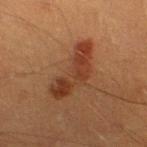Q: Was this lesion biopsied?
A: catalogued during a skin exam; not biopsied
Q: What lighting was used for the tile?
A: cross-polarized illumination
Q: Lesion location?
A: the left lower leg
Q: Automated lesion metrics?
A: a footprint of about 14 mm², a shape eccentricity near 0.95, and a shape-asymmetry score of about 0.5 (0 = symmetric); a mean CIELAB color near L≈31 a*≈20 b*≈27; border irregularity of about 6.5 on a 0–10 scale and a color-variation rating of about 4.5/10
Q: What kind of image is this?
A: ~15 mm tile from a whole-body skin photo
Q: What are the patient's age and sex?
A: male, about 40 years old
Q: What is the lesion's diameter?
A: about 7 mm